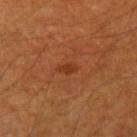imaging modality: total-body-photography crop, ~15 mm field of view
patient: male, about 60 years old
anatomic site: the arm